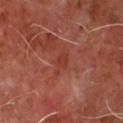Imaged during a routine full-body skin examination; the lesion was not biopsied and no histopathology is available. The patient is a male aged approximately 65. Automated image analysis of the tile measured a lesion area of about 3 mm², an outline eccentricity of about 0.9 (0 = round, 1 = elongated), and a symmetry-axis asymmetry near 0.35. Measured at roughly 3 mm in maximum diameter. This image is a 15 mm lesion crop taken from a total-body photograph. The lesion is located on the chest. The tile uses cross-polarized illumination.